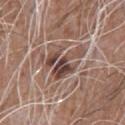Captured during whole-body skin photography for melanoma surveillance; the lesion was not biopsied. This is a white-light tile. Longest diameter approximately 5.5 mm. A 15 mm close-up tile from a total-body photography series done for melanoma screening. An algorithmic analysis of the crop reported a border-irregularity rating of about 9/10 and internal color variation of about 6.5 on a 0–10 scale. The software also gave a classifier nevus-likeness of about 10/100 and a lesion-detection confidence of about 60/100. A male subject, approximately 60 years of age. The lesion is located on the upper back.